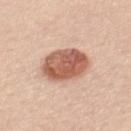A female subject, aged 43–47.
A close-up tile cropped from a whole-body skin photograph, about 15 mm across.
The lesion is on the mid back.
Imaged with white-light lighting.